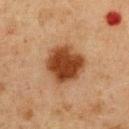This lesion was catalogued during total-body skin photography and was not selected for biopsy.
A roughly 15 mm field-of-view crop from a total-body skin photograph.
A male subject in their mid-70s.
An algorithmic analysis of the crop reported a footprint of about 16 mm², a shape eccentricity near 0.25, and two-axis asymmetry of about 0.15. It also reported a lesion color around L≈35 a*≈21 b*≈31 in CIELAB, a lesion–skin lightness drop of about 14, and a lesion-to-skin contrast of about 12.5 (normalized; higher = more distinct). The analysis additionally found a within-lesion color-variation index near 3.5/10 and radial color variation of about 1.
This is a cross-polarized tile.
From the chest.